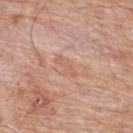follow-up: no biopsy performed (imaged during a skin exam); lighting: white-light illumination; location: the back; TBP lesion metrics: border irregularity of about 4 on a 0–10 scale, a within-lesion color-variation index near 1/10, and radial color variation of about 0.5; image source: ~15 mm crop, total-body skin-cancer survey; lesion diameter: ~3.5 mm (longest diameter); patient: male, aged around 80.